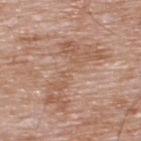The lesion was photographed on a routine skin check and not biopsied; there is no pathology result.